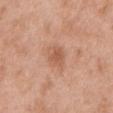tile lighting=white-light; location=the chest; image=total-body-photography crop, ~15 mm field of view; diameter=about 3 mm; subject=male, aged 48–52.